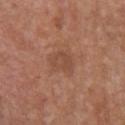Assessment: Imaged during a routine full-body skin examination; the lesion was not biopsied and no histopathology is available. Acquisition and patient details: A female patient about 75 years old. A roughly 15 mm field-of-view crop from a total-body skin photograph. Captured under white-light illumination. Longest diameter approximately 3.5 mm. From the chest. The total-body-photography lesion software estimated a lesion-detection confidence of about 100/100.